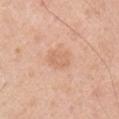Q: Is there a histopathology result?
A: imaged on a skin check; not biopsied
Q: Illumination type?
A: white-light illumination
Q: What kind of image is this?
A: total-body-photography crop, ~15 mm field of view
Q: What is the anatomic site?
A: the right upper arm
Q: How large is the lesion?
A: ≈3 mm
Q: What are the patient's age and sex?
A: male, aged approximately 55